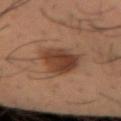A male patient, aged approximately 40.
A close-up tile cropped from a whole-body skin photograph, about 15 mm across.
On the left forearm.